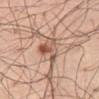This lesion was catalogued during total-body skin photography and was not selected for biopsy.
Automated image analysis of the tile measured a shape eccentricity near 0.75 and a symmetry-axis asymmetry near 0.55. The software also gave an average lesion color of about L≈56 a*≈21 b*≈29 (CIELAB), about 12 CIELAB-L* units darker than the surrounding skin, and a normalized lesion–skin contrast near 8. And it measured a classifier nevus-likeness of about 85/100.
Cropped from a total-body skin-imaging series; the visible field is about 15 mm.
On the abdomen.
A male subject aged 68–72.